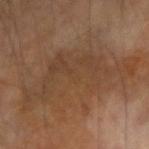Notes:
- notes: imaged on a skin check; not biopsied
- automated lesion analysis: a footprint of about 34 mm²; a lesion color around L≈39 a*≈16 b*≈29 in CIELAB, a lesion–skin lightness drop of about 7, and a normalized border contrast of about 6; border irregularity of about 9.5 on a 0–10 scale, a within-lesion color-variation index near 3/10, and a peripheral color-asymmetry measure near 1
- illumination: cross-polarized
- diameter: about 12.5 mm
- imaging modality: 15 mm crop, total-body photography
- subject: male, in their mid- to late 60s
- location: the left arm